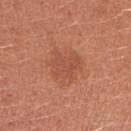Clinical impression: The lesion was photographed on a routine skin check and not biopsied; there is no pathology result.